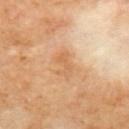Recorded during total-body skin imaging; not selected for excision or biopsy. A male patient, aged 68 to 72. A roughly 15 mm field-of-view crop from a total-body skin photograph. This is a cross-polarized tile. On the mid back. An algorithmic analysis of the crop reported a lesion area of about 5 mm², an eccentricity of roughly 0.85, and a shape-asymmetry score of about 0.4 (0 = symmetric). The software also gave a nevus-likeness score of about 0/100 and a lesion-detection confidence of about 100/100. The lesion's longest dimension is about 3.5 mm.